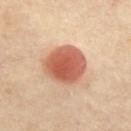Q: Was this lesion biopsied?
A: imaged on a skin check; not biopsied
Q: What is the imaging modality?
A: ~15 mm tile from a whole-body skin photo
Q: What is the lesion's diameter?
A: about 5 mm
Q: What is the anatomic site?
A: the chest
Q: Automated lesion metrics?
A: a footprint of about 18 mm² and a symmetry-axis asymmetry near 0.15